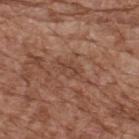follow-up: catalogued during a skin exam; not biopsied
patient: male, aged around 75
site: the upper back
imaging modality: 15 mm crop, total-body photography